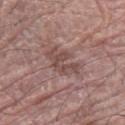| field | value |
|---|---|
| notes | total-body-photography surveillance lesion; no biopsy |
| patient | male, in their mid- to late 60s |
| diameter | ≈5 mm |
| automated lesion analysis | a lesion area of about 8.5 mm² and an eccentricity of roughly 0.9; a border-irregularity rating of about 6/10, internal color variation of about 3 on a 0–10 scale, and a peripheral color-asymmetry measure near 1 |
| lighting | white-light illumination |
| image | total-body-photography crop, ~15 mm field of view |
| location | the left forearm |